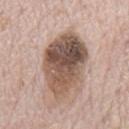Case summary:
* subject · male, approximately 70 years of age
* automated lesion analysis · about 18 CIELAB-L* units darker than the surrounding skin and a lesion-to-skin contrast of about 11.5 (normalized; higher = more distinct); border irregularity of about 2.5 on a 0–10 scale, a color-variation rating of about 9.5/10, and radial color variation of about 3.5
* lesion diameter · ≈8.5 mm
* tile lighting · white-light
* location · the mid back
* imaging modality · 15 mm crop, total-body photography